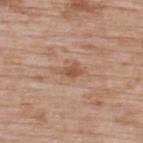| feature | finding |
|---|---|
| diameter | about 3 mm |
| tile lighting | white-light illumination |
| imaging modality | ~15 mm tile from a whole-body skin photo |
| patient | male, aged around 50 |
| location | the upper back |